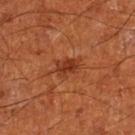follow-up: catalogued during a skin exam; not biopsied
automated lesion analysis: an area of roughly 5 mm², a shape eccentricity near 0.75, and a symmetry-axis asymmetry near 0.3; an average lesion color of about L≈35 a*≈28 b*≈34 (CIELAB); a lesion-detection confidence of about 100/100
image: total-body-photography crop, ~15 mm field of view
anatomic site: the leg
subject: male, aged around 65
tile lighting: cross-polarized illumination
diameter: about 3 mm